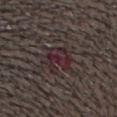* biopsy status · catalogued during a skin exam; not biopsied
* body site · the head or neck
* acquisition · ~15 mm crop, total-body skin-cancer survey
* patient · male, roughly 40 years of age
* automated metrics · border irregularity of about 4 on a 0–10 scale and a within-lesion color-variation index near 5.5/10
* lesion size · ~3 mm (longest diameter)
* lighting · white-light